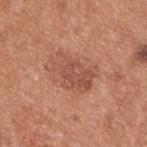Q: Was a biopsy performed?
A: no biopsy performed (imaged during a skin exam)
Q: What kind of image is this?
A: 15 mm crop, total-body photography
Q: What is the anatomic site?
A: the upper back
Q: What lighting was used for the tile?
A: white-light illumination
Q: Lesion size?
A: about 5.5 mm
Q: Patient demographics?
A: male, in their mid-50s
Q: What did automated image analysis measure?
A: an area of roughly 13 mm² and a shape-asymmetry score of about 0.35 (0 = symmetric); border irregularity of about 5 on a 0–10 scale, internal color variation of about 3 on a 0–10 scale, and radial color variation of about 1; a nevus-likeness score of about 30/100 and a lesion-detection confidence of about 100/100